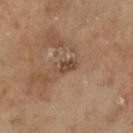| field | value |
|---|---|
| biopsy status | no biopsy performed (imaged during a skin exam) |
| acquisition | ~15 mm tile from a whole-body skin photo |
| location | the left lower leg |
| subject | male, aged 63 to 67 |